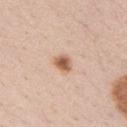biopsy status: catalogued during a skin exam; not biopsied
lesion size: about 2.5 mm
image source: total-body-photography crop, ~15 mm field of view
subject: female, approximately 40 years of age
illumination: white-light illumination
body site: the right upper arm
TBP lesion metrics: an area of roughly 4 mm², an eccentricity of roughly 0.6, and a shape-asymmetry score of about 0.25 (0 = symmetric); about 14 CIELAB-L* units darker than the surrounding skin; a border-irregularity index near 2/10 and a peripheral color-asymmetry measure near 1